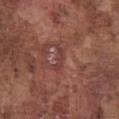From the chest. A 15 mm close-up tile from a total-body photography series done for melanoma screening. A male patient, in their mid- to late 70s.